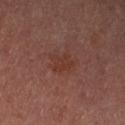notes=imaged on a skin check; not biopsied
subject=female
body site=the left lower leg
illumination=cross-polarized
image source=~15 mm crop, total-body skin-cancer survey
TBP lesion metrics=a lesion area of about 5.5 mm² and a shape eccentricity near 0.65; a border-irregularity index near 3/10 and internal color variation of about 1.5 on a 0–10 scale